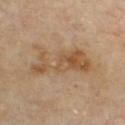<lesion>
<biopsy_status>not biopsied; imaged during a skin examination</biopsy_status>
<lesion_size>
  <long_diameter_mm_approx>7.5</long_diameter_mm_approx>
</lesion_size>
<image>
  <source>total-body photography crop</source>
  <field_of_view_mm>15</field_of_view_mm>
</image>
<site>chest</site>
<patient>
  <sex>male</sex>
  <age_approx>70</age_approx>
</patient>
</lesion>